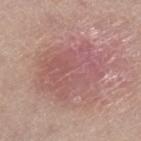Captured during whole-body skin photography for melanoma surveillance; the lesion was not biopsied. This image is a 15 mm lesion crop taken from a total-body photograph. Captured under white-light illumination. On the right lower leg. Approximately 7 mm at its widest. The total-body-photography lesion software estimated an area of roughly 29 mm², an eccentricity of roughly 0.65, and two-axis asymmetry of about 0.25. It also reported roughly 7 lightness units darker than nearby skin and a normalized lesion–skin contrast near 5. And it measured border irregularity of about 4 on a 0–10 scale, a within-lesion color-variation index near 5/10, and a peripheral color-asymmetry measure near 1.5. A female patient about 40 years old.